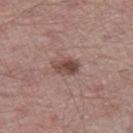Impression:
The lesion was photographed on a routine skin check and not biopsied; there is no pathology result.
Acquisition and patient details:
A 15 mm crop from a total-body photograph taken for skin-cancer surveillance. Automated tile analysis of the lesion measured roughly 12 lightness units darker than nearby skin and a normalized border contrast of about 9. The software also gave a border-irregularity rating of about 3/10 and a peripheral color-asymmetry measure near 2. It also reported an automated nevus-likeness rating near 90 out of 100 and a lesion-detection confidence of about 100/100. Imaged with white-light lighting. On the right thigh. Approximately 3 mm at its widest. A male patient in their mid- to late 60s.